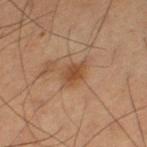The lesion was photographed on a routine skin check and not biopsied; there is no pathology result. A region of skin cropped from a whole-body photographic capture, roughly 15 mm wide. Located on the right thigh. A male subject, aged 58 to 62.